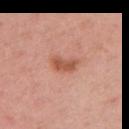subject: female, aged 33–37
lighting: white-light illumination
lesion diameter: ~3.5 mm (longest diameter)
acquisition: 15 mm crop, total-body photography
site: the left upper arm
image-analysis metrics: a lesion color around L≈55 a*≈26 b*≈32 in CIELAB, about 11 CIELAB-L* units darker than the surrounding skin, and a normalized border contrast of about 7.5; a lesion-detection confidence of about 100/100